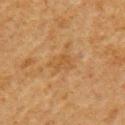This lesion was catalogued during total-body skin photography and was not selected for biopsy.
Automated image analysis of the tile measured a lesion area of about 4 mm² and two-axis asymmetry of about 0.25. It also reported an average lesion color of about L≈41 a*≈16 b*≈33 (CIELAB) and a lesion–skin lightness drop of about 5.
The lesion is located on the left upper arm.
Longest diameter approximately 2.5 mm.
A male patient in their 80s.
Captured under cross-polarized illumination.
A roughly 15 mm field-of-view crop from a total-body skin photograph.